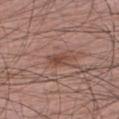notes: no biopsy performed (imaged during a skin exam)
image: 15 mm crop, total-body photography
subject: male, approximately 75 years of age
location: the arm
lighting: white-light